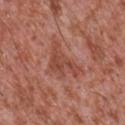– notes · total-body-photography surveillance lesion; no biopsy
– location · the front of the torso
– lesion size · ~5 mm (longest diameter)
– tile lighting · white-light
– patient · male, aged approximately 45
– image source · 15 mm crop, total-body photography